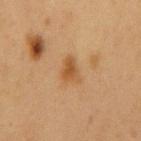notes: no biopsy performed (imaged during a skin exam) | illumination: cross-polarized illumination | automated lesion analysis: a mean CIELAB color near L≈47 a*≈20 b*≈37, roughly 8 lightness units darker than nearby skin, and a lesion-to-skin contrast of about 7.5 (normalized; higher = more distinct) | site: the chest | patient: male, aged around 55 | image: ~15 mm crop, total-body skin-cancer survey | size: ≈3 mm.